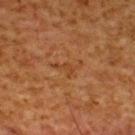The lesion was tiled from a total-body skin photograph and was not biopsied. A close-up tile cropped from a whole-body skin photograph, about 15 mm across. The subject is a male aged around 60. An algorithmic analysis of the crop reported an eccentricity of roughly 0.85 and a shape-asymmetry score of about 0.6 (0 = symmetric). The analysis additionally found a mean CIELAB color near L≈36 a*≈21 b*≈33, roughly 5 lightness units darker than nearby skin, and a normalized lesion–skin contrast near 5. The analysis additionally found internal color variation of about 0 on a 0–10 scale and a peripheral color-asymmetry measure near 0. Approximately 3.5 mm at its widest. The lesion is located on the upper back. This is a cross-polarized tile.